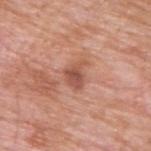Q: What is the lesion's diameter?
A: ≈3.5 mm
Q: Where on the body is the lesion?
A: the back
Q: Patient demographics?
A: male, about 60 years old
Q: How was this image acquired?
A: ~15 mm tile from a whole-body skin photo
Q: How was the tile lit?
A: white-light
Q: Automated lesion metrics?
A: a border-irregularity rating of about 4/10 and a within-lesion color-variation index near 3/10; a detector confidence of about 100 out of 100 that the crop contains a lesion